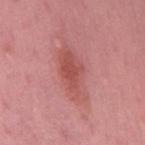Impression:
This lesion was catalogued during total-body skin photography and was not selected for biopsy.
Image and clinical context:
The subject is a male aged 53–57. Captured under white-light illumination. From the mid back. This image is a 15 mm lesion crop taken from a total-body photograph. The recorded lesion diameter is about 7.5 mm. The total-body-photography lesion software estimated a mean CIELAB color near L≈53 a*≈30 b*≈25, about 9 CIELAB-L* units darker than the surrounding skin, and a lesion-to-skin contrast of about 6.5 (normalized; higher = more distinct).